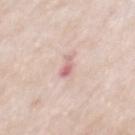This lesion was catalogued during total-body skin photography and was not selected for biopsy. A male subject, aged 63–67. A 15 mm close-up tile from a total-body photography series done for melanoma screening. Captured under white-light illumination. The lesion is on the mid back.